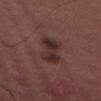Clinical impression: Imaged during a routine full-body skin examination; the lesion was not biopsied and no histopathology is available. Clinical summary: A male subject approximately 35 years of age. This image is a 15 mm lesion crop taken from a total-body photograph. From the front of the torso. Measured at roughly 4.5 mm in maximum diameter.